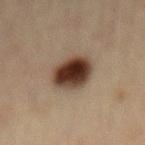follow-up — catalogued during a skin exam; not biopsied | lesion size — about 4.5 mm | TBP lesion metrics — a symmetry-axis asymmetry near 0.1; a mean CIELAB color near L≈32 a*≈14 b*≈22, a lesion–skin lightness drop of about 17, and a normalized lesion–skin contrast near 14.5; internal color variation of about 7 on a 0–10 scale and a peripheral color-asymmetry measure near 2 | patient — male, roughly 45 years of age | anatomic site — the mid back | image — total-body-photography crop, ~15 mm field of view.